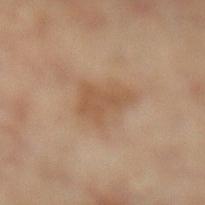Recorded during total-body skin imaging; not selected for excision or biopsy.
A 15 mm close-up tile from a total-body photography series done for melanoma screening.
Measured at roughly 4.5 mm in maximum diameter.
The tile uses cross-polarized illumination.
From the left lower leg.
An algorithmic analysis of the crop reported peripheral color asymmetry of about 1. The software also gave a nevus-likeness score of about 0/100 and lesion-presence confidence of about 100/100.
A female patient, aged 58 to 62.